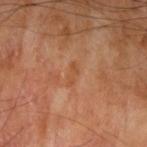<record>
  <biopsy_status>not biopsied; imaged during a skin examination</biopsy_status>
  <image>
    <source>total-body photography crop</source>
    <field_of_view_mm>15</field_of_view_mm>
  </image>
  <site>left upper arm</site>
  <patient>
    <sex>male</sex>
    <age_approx>65</age_approx>
  </patient>
</record>